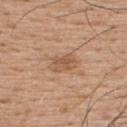Q: Was a biopsy performed?
A: total-body-photography surveillance lesion; no biopsy
Q: Where on the body is the lesion?
A: the upper back
Q: What did automated image analysis measure?
A: roughly 8 lightness units darker than nearby skin and a normalized lesion–skin contrast near 6
Q: Who is the patient?
A: male, roughly 55 years of age
Q: What kind of image is this?
A: ~15 mm tile from a whole-body skin photo